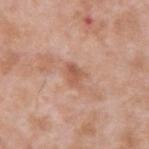Image and clinical context: About 3 mm across. The lesion is located on the back. A region of skin cropped from a whole-body photographic capture, roughly 15 mm wide. A male patient, aged 33–37. This is a white-light tile.